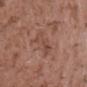Impression: Captured during whole-body skin photography for melanoma surveillance; the lesion was not biopsied. Image and clinical context: Located on the chest. A male patient aged 43–47. Captured under white-light illumination. A 15 mm crop from a total-body photograph taken for skin-cancer surveillance.